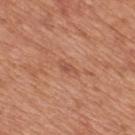{"automated_metrics": {"vs_skin_contrast_norm": 5.5, "peripheral_color_asymmetry": 0.0, "nevus_likeness_0_100": 0}, "patient": {"sex": "male", "age_approx": 65}, "image": {"source": "total-body photography crop", "field_of_view_mm": 15}, "site": "mid back"}